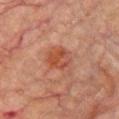Captured during whole-body skin photography for melanoma surveillance; the lesion was not biopsied. On the chest. Measured at roughly 3.5 mm in maximum diameter. A 15 mm crop from a total-body photograph taken for skin-cancer surveillance. The tile uses cross-polarized illumination. A male patient, aged around 65.